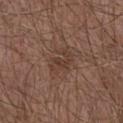This lesion was catalogued during total-body skin photography and was not selected for biopsy. Cropped from a whole-body photographic skin survey; the tile spans about 15 mm. From the chest. The tile uses white-light illumination. A male subject, in their mid- to late 60s. Automated tile analysis of the lesion measured a mean CIELAB color near L≈38 a*≈18 b*≈24 and a lesion–skin lightness drop of about 6. It also reported border irregularity of about 5 on a 0–10 scale, internal color variation of about 2 on a 0–10 scale, and peripheral color asymmetry of about 0.5. Measured at roughly 3.5 mm in maximum diameter.